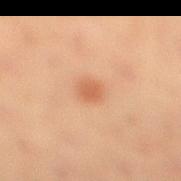Clinical impression: Recorded during total-body skin imaging; not selected for excision or biopsy. Acquisition and patient details: A 15 mm close-up extracted from a 3D total-body photography capture. Longest diameter approximately 2.5 mm. The lesion is located on the right lower leg. A female subject approximately 20 years of age. Captured under cross-polarized illumination.